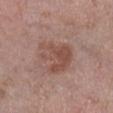biopsy status: total-body-photography surveillance lesion; no biopsy | image: 15 mm crop, total-body photography | patient: male, in their mid-50s | lesion diameter: ≈4.5 mm | location: the right lower leg | tile lighting: white-light illumination.